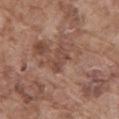- follow-up — total-body-photography surveillance lesion; no biopsy
- size — ~3.5 mm (longest diameter)
- tile lighting — white-light
- location — the mid back
- acquisition — 15 mm crop, total-body photography
- patient — male, in their mid- to late 70s